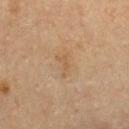This lesion was catalogued during total-body skin photography and was not selected for biopsy. A male patient approximately 60 years of age. A 15 mm close-up extracted from a 3D total-body photography capture. Longest diameter approximately 3 mm. On the front of the torso.